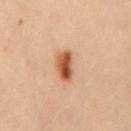Findings:
• subject — female, aged approximately 30
• location — the lower back
• illumination — cross-polarized illumination
• acquisition — ~15 mm crop, total-body skin-cancer survey